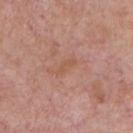{"biopsy_status": "not biopsied; imaged during a skin examination", "patient": {"sex": "male", "age_approx": 75}, "image": {"source": "total-body photography crop", "field_of_view_mm": 15}, "site": "chest"}